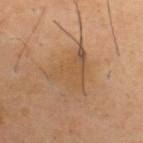workup: total-body-photography surveillance lesion; no biopsy
size: ~6 mm (longest diameter)
illumination: cross-polarized illumination
patient: male, roughly 40 years of age
site: the upper back
automated lesion analysis: a lesion color around L≈52 a*≈18 b*≈34 in CIELAB, a lesion–skin lightness drop of about 7, and a lesion-to-skin contrast of about 5 (normalized; higher = more distinct); border irregularity of about 6 on a 0–10 scale, a color-variation rating of about 4/10, and peripheral color asymmetry of about 1.5
acquisition: ~15 mm crop, total-body skin-cancer survey